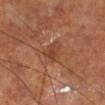Imaged during a routine full-body skin examination; the lesion was not biopsied and no histopathology is available.
Measured at roughly 3 mm in maximum diameter.
A roughly 15 mm field-of-view crop from a total-body skin photograph.
The subject is a male aged around 70.
Captured under cross-polarized illumination.
From the leg.